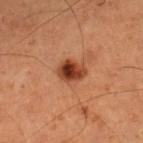notes: imaged on a skin check; not biopsied | image-analysis metrics: a footprint of about 6.5 mm², an outline eccentricity of about 0.6 (0 = round, 1 = elongated), and a shape-asymmetry score of about 0.2 (0 = symmetric); an automated nevus-likeness rating near 100 out of 100 and lesion-presence confidence of about 100/100 | imaging modality: total-body-photography crop, ~15 mm field of view | patient: male, aged around 60 | site: the right lower leg | illumination: cross-polarized | lesion diameter: about 3 mm.